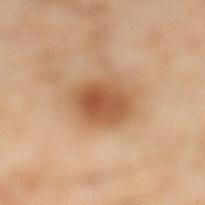Acquisition and patient details:
A male subject aged around 55. From the left lower leg. Approximately 4.5 mm at its widest. A region of skin cropped from a whole-body photographic capture, roughly 15 mm wide. The tile uses cross-polarized illumination.